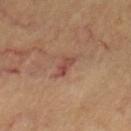Background: Measured at roughly 3 mm in maximum diameter. A 15 mm close-up tile from a total-body photography series done for melanoma screening. The patient is a female aged around 60. From the left thigh.